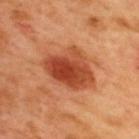Imaged during a routine full-body skin examination; the lesion was not biopsied and no histopathology is available.
Automated tile analysis of the lesion measured a footprint of about 19 mm², a shape eccentricity near 0.7, and a symmetry-axis asymmetry near 0.25. It also reported a color-variation rating of about 7/10. And it measured a nevus-likeness score of about 100/100 and a lesion-detection confidence of about 100/100.
The tile uses cross-polarized illumination.
Located on the upper back.
About 6 mm across.
A 15 mm close-up tile from a total-body photography series done for melanoma screening.
The subject is a male aged approximately 50.